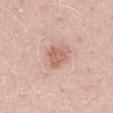biopsy status = catalogued during a skin exam; not biopsied
patient = male, about 50 years old
body site = the right thigh
image = ~15 mm tile from a whole-body skin photo
tile lighting = white-light